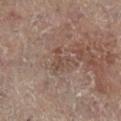Findings:
– biopsy status: imaged on a skin check; not biopsied
– automated lesion analysis: an average lesion color of about L≈41 a*≈15 b*≈22 (CIELAB) and a lesion–skin lightness drop of about 6; a border-irregularity index near 6/10 and a peripheral color-asymmetry measure near 0.5
– subject: female, aged approximately 80
– lesion diameter: about 3 mm
– body site: the left leg
– imaging modality: 15 mm crop, total-body photography
– illumination: cross-polarized